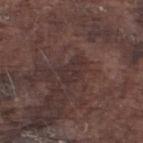Assessment: Imaged during a routine full-body skin examination; the lesion was not biopsied and no histopathology is available. Background: The lesion-visualizer software estimated a lesion area of about 5.5 mm² and a symmetry-axis asymmetry near 0.4. The analysis additionally found a lesion color around L≈30 a*≈15 b*≈16 in CIELAB and a normalized lesion–skin contrast near 5. The software also gave a nevus-likeness score of about 0/100 and a lesion-detection confidence of about 75/100. The lesion is located on the left lower leg. Captured under white-light illumination. A roughly 15 mm field-of-view crop from a total-body skin photograph. Longest diameter approximately 3 mm. A male subject, in their mid- to late 70s.